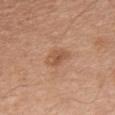Imaged during a routine full-body skin examination; the lesion was not biopsied and no histopathology is available. This is a white-light tile. The recorded lesion diameter is about 3 mm. The lesion is on the chest. The subject is a male approximately 65 years of age. Cropped from a whole-body photographic skin survey; the tile spans about 15 mm.